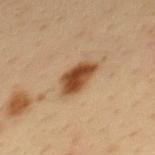Q: Is there a histopathology result?
A: total-body-photography surveillance lesion; no biopsy
Q: What is the anatomic site?
A: the mid back
Q: What is the imaging modality?
A: total-body-photography crop, ~15 mm field of view
Q: What did automated image analysis measure?
A: an automated nevus-likeness rating near 100 out of 100
Q: Illumination type?
A: cross-polarized illumination
Q: Patient demographics?
A: male, in their mid-30s
Q: What is the lesion's diameter?
A: about 4.5 mm